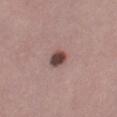* follow-up · no biopsy performed (imaged during a skin exam)
* patient · female, in their mid- to late 50s
* illumination · white-light
* site · the right thigh
* image · 15 mm crop, total-body photography
* diameter · ~2.5 mm (longest diameter)